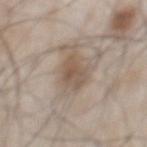Impression: The lesion was photographed on a routine skin check and not biopsied; there is no pathology result. Context: From the chest. A lesion tile, about 15 mm wide, cut from a 3D total-body photograph. The patient is a male aged 53–57. Longest diameter approximately 4 mm. The lesion-visualizer software estimated a classifier nevus-likeness of about 30/100 and a lesion-detection confidence of about 95/100.